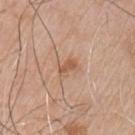Notes:
– biopsy status · no biopsy performed (imaged during a skin exam)
– body site · the chest
– imaging modality · ~15 mm tile from a whole-body skin photo
– patient · male, aged 73 to 77